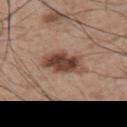Clinical impression:
Captured during whole-body skin photography for melanoma surveillance; the lesion was not biopsied.
Background:
A region of skin cropped from a whole-body photographic capture, roughly 15 mm wide. An algorithmic analysis of the crop reported an area of roughly 11 mm², an eccentricity of roughly 0.85, and two-axis asymmetry of about 0.25. The software also gave a lesion–skin lightness drop of about 15 and a normalized lesion–skin contrast near 11. The subject is a male aged 63–67. The lesion is on the upper back.